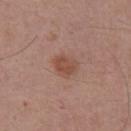  image:
    source: total-body photography crop
    field_of_view_mm: 15
  site: chest
  lesion_size:
    long_diameter_mm_approx: 3.0
  patient:
    sex: male
    age_approx: 55